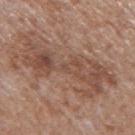A 15 mm crop from a total-body photograph taken for skin-cancer surveillance. The subject is a male aged 63 to 67. On the mid back.